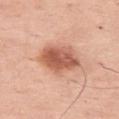biopsy status: total-body-photography surveillance lesion; no biopsy | patient: female, aged around 65 | anatomic site: the left thigh | image: 15 mm crop, total-body photography.